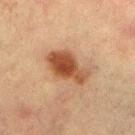workup: imaged on a skin check; not biopsied | imaging modality: total-body-photography crop, ~15 mm field of view | image-analysis metrics: a footprint of about 13 mm², an eccentricity of roughly 0.85, and two-axis asymmetry of about 0.25; an average lesion color of about L≈40 a*≈20 b*≈30 (CIELAB), roughly 13 lightness units darker than nearby skin, and a lesion-to-skin contrast of about 10.5 (normalized; higher = more distinct) | anatomic site: the left lower leg | subject: female, roughly 55 years of age | illumination: cross-polarized illumination.